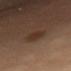– biopsy status: catalogued during a skin exam; not biopsied
– imaging modality: ~15 mm tile from a whole-body skin photo
– patient: female, approximately 55 years of age
– anatomic site: the chest
– automated metrics: an area of roughly 5.5 mm², an eccentricity of roughly 0.85, and a shape-asymmetry score of about 0.15 (0 = symmetric); a border-irregularity rating of about 2/10 and a within-lesion color-variation index near 2.5/10
– size: ~4 mm (longest diameter)
– illumination: cross-polarized